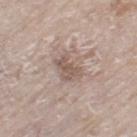No biopsy was performed on this lesion — it was imaged during a full skin examination and was not determined to be concerning. From the leg. Imaged with white-light lighting. This image is a 15 mm lesion crop taken from a total-body photograph. The patient is a female aged 73 to 77. The recorded lesion diameter is about 3.5 mm. Automated tile analysis of the lesion measured an area of roughly 7.5 mm², a shape eccentricity near 0.7, and two-axis asymmetry of about 0.3. It also reported an average lesion color of about L≈56 a*≈15 b*≈22 (CIELAB), about 9 CIELAB-L* units darker than the surrounding skin, and a lesion-to-skin contrast of about 6.5 (normalized; higher = more distinct).